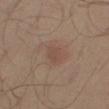Case summary:
* follow-up: imaged on a skin check; not biopsied
* patient: male, aged around 55
* imaging modality: 15 mm crop, total-body photography
* lighting: cross-polarized illumination
* site: the right thigh
* lesion diameter: ~2.5 mm (longest diameter)